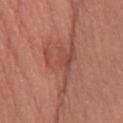Recorded during total-body skin imaging; not selected for excision or biopsy.
A 15 mm close-up tile from a total-body photography series done for melanoma screening.
Located on the chest.
Measured at roughly 10.5 mm in maximum diameter.
The subject is a female roughly 70 years of age.
Automated image analysis of the tile measured an area of roughly 18 mm², a shape eccentricity near 0.9, and two-axis asymmetry of about 0.65.